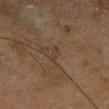biopsy status: no biopsy performed (imaged during a skin exam) | anatomic site: the right lower leg | imaging modality: ~15 mm tile from a whole-body skin photo | lighting: cross-polarized | subject: male, in their mid- to late 60s | lesion size: ~2.5 mm (longest diameter) | automated metrics: a mean CIELAB color near L≈39 a*≈14 b*≈27, roughly 5 lightness units darker than nearby skin, and a normalized border contrast of about 4.5; border irregularity of about 4 on a 0–10 scale and peripheral color asymmetry of about 0; a classifier nevus-likeness of about 0/100 and a detector confidence of about 65 out of 100 that the crop contains a lesion.